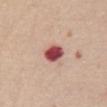Recorded during total-body skin imaging; not selected for excision or biopsy. The patient is a female about 70 years old. A 15 mm crop from a total-body photograph taken for skin-cancer surveillance. From the front of the torso.